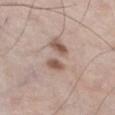Captured during whole-body skin photography for melanoma surveillance; the lesion was not biopsied. This is a white-light tile. A lesion tile, about 15 mm wide, cut from a 3D total-body photograph. The lesion is on the left lower leg. Automated tile analysis of the lesion measured an area of roughly 16 mm², an outline eccentricity of about 0.85 (0 = round, 1 = elongated), and a shape-asymmetry score of about 0.35 (0 = symmetric). It also reported a border-irregularity rating of about 5.5/10, internal color variation of about 9 on a 0–10 scale, and peripheral color asymmetry of about 3. A male patient, in their 60s. Measured at roughly 7 mm in maximum diameter.